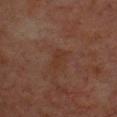A male patient, in their 60s. A 15 mm close-up tile from a total-body photography series done for melanoma screening. On the upper back.